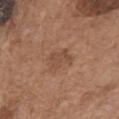Impression: This lesion was catalogued during total-body skin photography and was not selected for biopsy. Background: Imaged with white-light lighting. A male patient, in their mid- to late 70s. The recorded lesion diameter is about 3 mm. On the chest. An algorithmic analysis of the crop reported an area of roughly 5.5 mm², an outline eccentricity of about 0.7 (0 = round, 1 = elongated), and a symmetry-axis asymmetry near 0.35. And it measured a border-irregularity index near 4/10, a within-lesion color-variation index near 1.5/10, and a peripheral color-asymmetry measure near 0.5. And it measured a nevus-likeness score of about 0/100 and a detector confidence of about 100 out of 100 that the crop contains a lesion. A roughly 15 mm field-of-view crop from a total-body skin photograph.